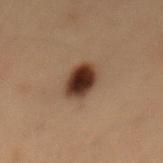follow-up = total-body-photography surveillance lesion; no biopsy | image source = ~15 mm crop, total-body skin-cancer survey | tile lighting = cross-polarized | lesion diameter = ≈4.5 mm | subject = male, roughly 55 years of age | site = the mid back.